Located on the abdomen. A 15 mm close-up extracted from a 3D total-body photography capture. Imaged with white-light lighting. Automated image analysis of the tile measured a lesion area of about 1 mm² and an outline eccentricity of about 0.85 (0 = round, 1 = elongated). The software also gave an average lesion color of about L≈44 a*≈18 b*≈23 (CIELAB), about 6 CIELAB-L* units darker than the surrounding skin, and a normalized lesion–skin contrast near 5.5. And it measured a classifier nevus-likeness of about 0/100 and a lesion-detection confidence of about 0/100. A male subject, aged approximately 70. Longest diameter approximately 1.5 mm.
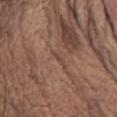Q: What did pathology find?
A: a seborrheic keratosis (benign)